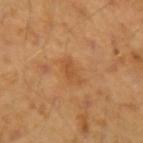Q: Is there a histopathology result?
A: no biopsy performed (imaged during a skin exam)
Q: What is the anatomic site?
A: the left forearm
Q: Lesion size?
A: ≈3.5 mm
Q: Patient demographics?
A: male, aged 43 to 47
Q: How was this image acquired?
A: ~15 mm crop, total-body skin-cancer survey
Q: Illumination type?
A: cross-polarized illumination
Q: What did automated image analysis measure?
A: a normalized border contrast of about 5; internal color variation of about 2.5 on a 0–10 scale and peripheral color asymmetry of about 1